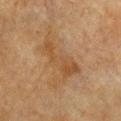Case summary:
* lesion size · ~6 mm (longest diameter)
* TBP lesion metrics · a lesion area of about 10 mm² and a shape eccentricity near 0.95; an average lesion color of about L≈40 a*≈16 b*≈31 (CIELAB), about 6 CIELAB-L* units darker than the surrounding skin, and a lesion-to-skin contrast of about 6 (normalized; higher = more distinct); an automated nevus-likeness rating near 0 out of 100 and a detector confidence of about 100 out of 100 that the crop contains a lesion
* patient · female, aged 53 to 57
* anatomic site · the chest
* imaging modality · ~15 mm tile from a whole-body skin photo
* illumination · cross-polarized